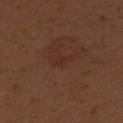Notes:
• biopsy status — catalogued during a skin exam; not biopsied
• illumination — white-light illumination
• diameter — ~1.5 mm (longest diameter)
• image source — 15 mm crop, total-body photography
• subject — female, in their 30s
• location — the right upper arm